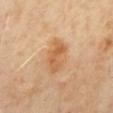- biopsy status · catalogued during a skin exam; not biopsied
- lesion diameter · ≈4 mm
- illumination · cross-polarized
- anatomic site · the mid back
- image source · ~15 mm tile from a whole-body skin photo
- TBP lesion metrics · a footprint of about 7.5 mm² and a symmetry-axis asymmetry near 0.3
- subject · male, roughly 65 years of age